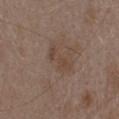<tbp_lesion>
  <biopsy_status>not biopsied; imaged during a skin examination</biopsy_status>
  <image>
    <source>total-body photography crop</source>
    <field_of_view_mm>15</field_of_view_mm>
  </image>
  <patient>
    <sex>female</sex>
    <age_approx>45</age_approx>
  </patient>
  <site>head or neck</site>
</tbp_lesion>